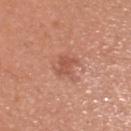Q: Was this lesion biopsied?
A: catalogued during a skin exam; not biopsied
Q: How was the tile lit?
A: white-light
Q: Automated lesion metrics?
A: a footprint of about 3.5 mm², an outline eccentricity of about 0.8 (0 = round, 1 = elongated), and a shape-asymmetry score of about 0.35 (0 = symmetric); border irregularity of about 3.5 on a 0–10 scale, a within-lesion color-variation index near 1/10, and a peripheral color-asymmetry measure near 0.5
Q: How large is the lesion?
A: ≈2.5 mm
Q: What is the imaging modality?
A: 15 mm crop, total-body photography
Q: Lesion location?
A: the head or neck
Q: Who is the patient?
A: male, aged 38–42